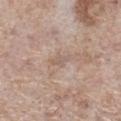{
  "biopsy_status": "not biopsied; imaged during a skin examination",
  "lighting": "white-light",
  "patient": {
    "sex": "female",
    "age_approx": 55
  },
  "lesion_size": {
    "long_diameter_mm_approx": 2.5
  },
  "site": "leg",
  "image": {
    "source": "total-body photography crop",
    "field_of_view_mm": 15
  }
}